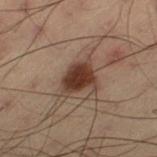workup = imaged on a skin check; not biopsied
subject = male, aged approximately 55
illumination = cross-polarized illumination
lesion size = ≈3.5 mm
imaging modality = ~15 mm crop, total-body skin-cancer survey
body site = the left thigh